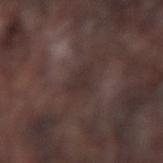workup: no biopsy performed (imaged during a skin exam) | imaging modality: total-body-photography crop, ~15 mm field of view | subject: male, aged 73 to 77 | size: ~2.5 mm (longest diameter) | tile lighting: white-light | location: the left forearm | automated lesion analysis: an average lesion color of about L≈29 a*≈13 b*≈15 (CIELAB), roughly 5 lightness units darker than nearby skin, and a normalized border contrast of about 5.5; radial color variation of about 0.5.